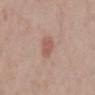workup: total-body-photography surveillance lesion; no biopsy | lesion size: about 3 mm | image source: 15 mm crop, total-body photography | subject: male, aged approximately 70 | body site: the mid back | tile lighting: white-light illumination.